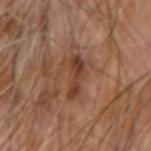From the left forearm.
A male subject, in their mid- to late 60s.
The lesion's longest dimension is about 4.5 mm.
A 15 mm close-up tile from a total-body photography series done for melanoma screening.